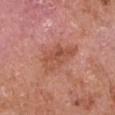lesion_size:
  long_diameter_mm_approx: 5.5
lighting: white-light
image:
  source: total-body photography crop
  field_of_view_mm: 15
site: left upper arm
patient:
  sex: male
  age_approx: 65